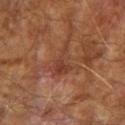* follow-up: total-body-photography surveillance lesion; no biopsy
* TBP lesion metrics: a footprint of about 6.5 mm² and a shape-asymmetry score of about 0.55 (0 = symmetric); a mean CIELAB color near L≈38 a*≈23 b*≈29, a lesion–skin lightness drop of about 7, and a normalized border contrast of about 6; border irregularity of about 7 on a 0–10 scale; a lesion-detection confidence of about 50/100
* lighting: cross-polarized illumination
* location: the arm
* patient: male, roughly 65 years of age
* imaging modality: ~15 mm tile from a whole-body skin photo
* diameter: ~5 mm (longest diameter)